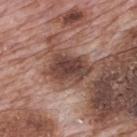Recorded during total-body skin imaging; not selected for excision or biopsy. Located on the mid back. A region of skin cropped from a whole-body photographic capture, roughly 15 mm wide. The subject is a male roughly 70 years of age.